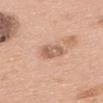The lesion was tiled from a total-body skin photograph and was not biopsied. Cropped from a whole-body photographic skin survey; the tile spans about 15 mm. On the upper back. The subject is a female aged 58–62. Automated tile analysis of the lesion measured a lesion color around L≈60 a*≈21 b*≈30 in CIELAB and a normalized border contrast of about 6.5. The analysis additionally found border irregularity of about 2 on a 0–10 scale. The recorded lesion diameter is about 3 mm. Captured under white-light illumination.